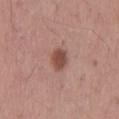Impression: Captured during whole-body skin photography for melanoma surveillance; the lesion was not biopsied. Context: A roughly 15 mm field-of-view crop from a total-body skin photograph. Located on the back. A male patient, aged around 55.